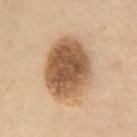Captured during whole-body skin photography for melanoma surveillance; the lesion was not biopsied. On the chest. A female patient, about 55 years old. The lesion-visualizer software estimated a shape eccentricity near 0.65 and two-axis asymmetry of about 0.1. Captured under cross-polarized illumination. A 15 mm crop from a total-body photograph taken for skin-cancer surveillance.